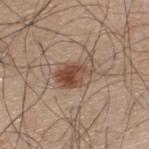{"biopsy_status": "not biopsied; imaged during a skin examination", "lighting": "white-light", "site": "upper back", "lesion_size": {"long_diameter_mm_approx": 4.5}, "patient": {"sex": "male", "age_approx": 45}, "image": {"source": "total-body photography crop", "field_of_view_mm": 15}}